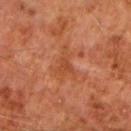The lesion was photographed on a routine skin check and not biopsied; there is no pathology result.